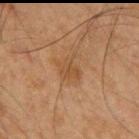Impression: The lesion was tiled from a total-body skin photograph and was not biopsied. Image and clinical context: A 15 mm close-up tile from a total-body photography series done for melanoma screening. The recorded lesion diameter is about 3 mm. The lesion is located on the mid back. The subject is a male in their mid- to late 60s.